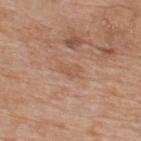<case>
<biopsy_status>not biopsied; imaged during a skin examination</biopsy_status>
<automated_metrics>
  <area_mm2_approx>2.5</area_mm2_approx>
  <eccentricity>0.85</eccentricity>
  <shape_asymmetry>0.4</shape_asymmetry>
  <cielab_L>55</cielab_L>
  <cielab_a>20</cielab_a>
  <cielab_b>31</cielab_b>
  <vs_skin_darker_L>6.0</vs_skin_darker_L>
  <vs_skin_contrast_norm>4.5</vs_skin_contrast_norm>
  <border_irregularity_0_10>4.0</border_irregularity_0_10>
  <color_variation_0_10>0.0</color_variation_0_10>
  <peripheral_color_asymmetry>0.0</peripheral_color_asymmetry>
  <nevus_likeness_0_100>0</nevus_likeness_0_100>
  <lesion_detection_confidence_0_100>95</lesion_detection_confidence_0_100>
</automated_metrics>
<site>upper back</site>
<lesion_size>
  <long_diameter_mm_approx>2.5</long_diameter_mm_approx>
</lesion_size>
<patient>
  <sex>male</sex>
  <age_approx>60</age_approx>
</patient>
<image>
  <source>total-body photography crop</source>
  <field_of_view_mm>15</field_of_view_mm>
</image>
</case>